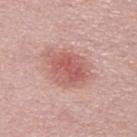Imaged during a routine full-body skin examination; the lesion was not biopsied and no histopathology is available. Measured at roughly 6 mm in maximum diameter. The patient is a male in their 30s. Captured under white-light illumination. Cropped from a total-body skin-imaging series; the visible field is about 15 mm.